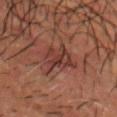{
  "biopsy_status": "not biopsied; imaged during a skin examination",
  "automated_metrics": {
    "cielab_L": 27,
    "cielab_a": 18,
    "cielab_b": 19,
    "vs_skin_darker_L": 6.0,
    "vs_skin_contrast_norm": 6.5,
    "nevus_likeness_0_100": 5,
    "lesion_detection_confidence_0_100": 60
  },
  "image": {
    "source": "total-body photography crop",
    "field_of_view_mm": 15
  },
  "lighting": "cross-polarized",
  "patient": {
    "sex": "male",
    "age_approx": 50
  },
  "site": "head or neck"
}